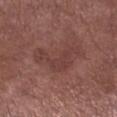Imaged during a routine full-body skin examination; the lesion was not biopsied and no histopathology is available.
An algorithmic analysis of the crop reported a lesion area of about 12 mm², an outline eccentricity of about 0.85 (0 = round, 1 = elongated), and a shape-asymmetry score of about 0.7 (0 = symmetric).
The recorded lesion diameter is about 6.5 mm.
A 15 mm crop from a total-body photograph taken for skin-cancer surveillance.
A male subject about 55 years old.
The lesion is on the right lower leg.